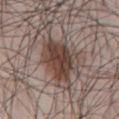Recorded during total-body skin imaging; not selected for excision or biopsy. The lesion is located on the abdomen. A male patient in their mid-40s. The tile uses white-light illumination. A close-up tile cropped from a whole-body skin photograph, about 15 mm across.